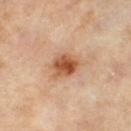Clinical impression:
This lesion was catalogued during total-body skin photography and was not selected for biopsy.
Image and clinical context:
An algorithmic analysis of the crop reported an area of roughly 7.5 mm², an eccentricity of roughly 0.2, and two-axis asymmetry of about 0.2. It also reported a mean CIELAB color near L≈52 a*≈23 b*≈36, about 13 CIELAB-L* units darker than the surrounding skin, and a normalized border contrast of about 9.5. The analysis additionally found a border-irregularity rating of about 2/10, a color-variation rating of about 5.5/10, and a peripheral color-asymmetry measure near 2. The subject is a female in their 50s. A roughly 15 mm field-of-view crop from a total-body skin photograph. Captured under cross-polarized illumination. From the left thigh.